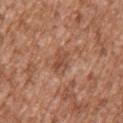Clinical impression:
Imaged during a routine full-body skin examination; the lesion was not biopsied and no histopathology is available.
Acquisition and patient details:
The tile uses white-light illumination. The total-body-photography lesion software estimated a lesion area of about 5.5 mm², an eccentricity of roughly 0.85, and a symmetry-axis asymmetry near 0.3. The software also gave a border-irregularity index near 3/10 and radial color variation of about 1.5. The software also gave a lesion-detection confidence of about 100/100. A male patient, aged around 45. Approximately 3.5 mm at its widest. A region of skin cropped from a whole-body photographic capture, roughly 15 mm wide. On the arm.